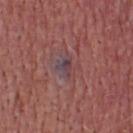Part of a total-body skin-imaging series; this lesion was reviewed on a skin check and was not flagged for biopsy. The tile uses white-light illumination. The subject is a male in their 60s. Cropped from a whole-body photographic skin survey; the tile spans about 15 mm. Automated tile analysis of the lesion measured a color-variation rating of about 4.5/10 and peripheral color asymmetry of about 1. The analysis additionally found a nevus-likeness score of about 0/100 and a detector confidence of about 100 out of 100 that the crop contains a lesion. The lesion is located on the head or neck.